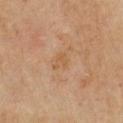Q: Was a biopsy performed?
A: total-body-photography surveillance lesion; no biopsy
Q: What is the imaging modality?
A: ~15 mm tile from a whole-body skin photo
Q: Lesion size?
A: ~2.5 mm (longest diameter)
Q: What lighting was used for the tile?
A: cross-polarized
Q: What is the anatomic site?
A: the front of the torso
Q: Who is the patient?
A: male, aged approximately 70
Q: What did automated image analysis measure?
A: a footprint of about 3 mm², a shape eccentricity near 0.7, and a symmetry-axis asymmetry near 0.45; a lesion color around L≈53 a*≈18 b*≈35 in CIELAB and about 5 CIELAB-L* units darker than the surrounding skin; a border-irregularity rating of about 5/10 and radial color variation of about 0.5; a classifier nevus-likeness of about 0/100 and a lesion-detection confidence of about 100/100